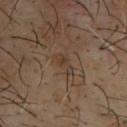{
  "biopsy_status": "not biopsied; imaged during a skin examination",
  "image": {
    "source": "total-body photography crop",
    "field_of_view_mm": 15
  },
  "lesion_size": {
    "long_diameter_mm_approx": 3.0
  },
  "lighting": "cross-polarized",
  "site": "back",
  "patient": {
    "sex": "male",
    "age_approx": 65
  }
}